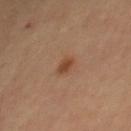<record>
<biopsy_status>not biopsied; imaged during a skin examination</biopsy_status>
<automated_metrics>
  <cielab_L>40</cielab_L>
  <cielab_a>20</cielab_a>
  <cielab_b>30</cielab_b>
  <vs_skin_darker_L>8.0</vs_skin_darker_L>
  <vs_skin_contrast_norm>7.5</vs_skin_contrast_norm>
</automated_metrics>
<image>
  <source>total-body photography crop</source>
  <field_of_view_mm>15</field_of_view_mm>
</image>
<site>mid back</site>
<patient>
  <sex>male</sex>
  <age_approx>65</age_approx>
</patient>
</record>